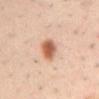Impression:
This lesion was catalogued during total-body skin photography and was not selected for biopsy.
Context:
The total-body-photography lesion software estimated an average lesion color of about L≈59 a*≈23 b*≈32 (CIELAB), about 15 CIELAB-L* units darker than the surrounding skin, and a lesion-to-skin contrast of about 10 (normalized; higher = more distinct). The software also gave internal color variation of about 5 on a 0–10 scale and peripheral color asymmetry of about 1.5. Approximately 4 mm at its widest. Cropped from a whole-body photographic skin survey; the tile spans about 15 mm. The tile uses cross-polarized illumination. From the back. A male patient, in their 40s.